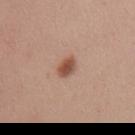<tbp_lesion>
<biopsy_status>not biopsied; imaged during a skin examination</biopsy_status>
<lesion_size>
  <long_diameter_mm_approx>3.0</long_diameter_mm_approx>
</lesion_size>
<patient>
  <sex>female</sex>
  <age_approx>25</age_approx>
</patient>
<site>mid back</site>
<lighting>white-light</lighting>
<image>
  <source>total-body photography crop</source>
  <field_of_view_mm>15</field_of_view_mm>
</image>
<automated_metrics>
  <cielab_L>52</cielab_L>
  <cielab_a>22</cielab_a>
  <cielab_b>29</cielab_b>
  <vs_skin_darker_L>12.0</vs_skin_darker_L>
  <vs_skin_contrast_norm>8.5</vs_skin_contrast_norm>
  <lesion_detection_confidence_0_100>100</lesion_detection_confidence_0_100>
</automated_metrics>
</tbp_lesion>